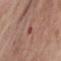{
  "biopsy_status": "not biopsied; imaged during a skin examination",
  "patient": {
    "sex": "female",
    "age_approx": 55
  },
  "automated_metrics": {
    "area_mm2_approx": 2.5,
    "eccentricity": 0.95,
    "shape_asymmetry": 0.35,
    "vs_skin_darker_L": 8.0
  },
  "site": "right lower leg",
  "image": {
    "source": "total-body photography crop",
    "field_of_view_mm": 15
  },
  "lesion_size": {
    "long_diameter_mm_approx": 3.0
  },
  "lighting": "cross-polarized"
}